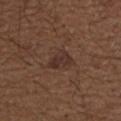This lesion was catalogued during total-body skin photography and was not selected for biopsy. This image is a 15 mm lesion crop taken from a total-body photograph. The lesion is on the right upper arm. A male subject, about 50 years old.